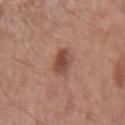Q: Is there a histopathology result?
A: total-body-photography surveillance lesion; no biopsy
Q: How was the tile lit?
A: white-light illumination
Q: What are the patient's age and sex?
A: female, aged around 50
Q: What did automated image analysis measure?
A: an area of roughly 6 mm² and an outline eccentricity of about 0.6 (0 = round, 1 = elongated); a border-irregularity index near 1.5/10, internal color variation of about 3.5 on a 0–10 scale, and radial color variation of about 1
Q: How was this image acquired?
A: ~15 mm crop, total-body skin-cancer survey
Q: Where on the body is the lesion?
A: the right forearm
Q: What is the lesion's diameter?
A: ≈3 mm